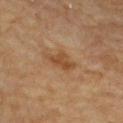Q: Is there a histopathology result?
A: catalogued during a skin exam; not biopsied
Q: How was this image acquired?
A: total-body-photography crop, ~15 mm field of view
Q: Who is the patient?
A: male, aged around 85 or older
Q: Automated lesion metrics?
A: a lesion area of about 5.5 mm² and two-axis asymmetry of about 0.3; a lesion color around L≈46 a*≈20 b*≈35 in CIELAB, a lesion–skin lightness drop of about 9, and a normalized border contrast of about 7.5; internal color variation of about 2 on a 0–10 scale and a peripheral color-asymmetry measure near 0.5
Q: What lighting was used for the tile?
A: cross-polarized illumination
Q: Where on the body is the lesion?
A: the back